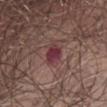| feature | finding |
|---|---|
| follow-up | imaged on a skin check; not biopsied |